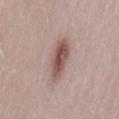notes: total-body-photography surveillance lesion; no biopsy | diameter: about 5 mm | image: ~15 mm tile from a whole-body skin photo | patient: female, in their 30s | anatomic site: the lower back | automated lesion analysis: a lesion color around L≈53 a*≈19 b*≈22 in CIELAB; an automated nevus-likeness rating near 95 out of 100 and lesion-presence confidence of about 100/100.